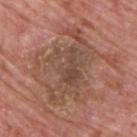Findings:
* notes · catalogued during a skin exam; not biopsied
* image · ~15 mm crop, total-body skin-cancer survey
* anatomic site · the upper back
* patient · male, approximately 75 years of age
* lesion diameter · about 9 mm